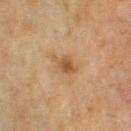<lesion>
<biopsy_status>not biopsied; imaged during a skin examination</biopsy_status>
<image>
  <source>total-body photography crop</source>
  <field_of_view_mm>15</field_of_view_mm>
</image>
<patient>
  <sex>female</sex>
  <age_approx>55</age_approx>
</patient>
<lighting>cross-polarized</lighting>
<site>left thigh</site>
</lesion>